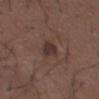Q: Was this lesion biopsied?
A: catalogued during a skin exam; not biopsied